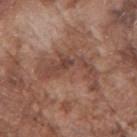Clinical impression: The lesion was photographed on a routine skin check and not biopsied; there is no pathology result. Context: The lesion's longest dimension is about 7 mm. This is a white-light tile. A male patient, aged approximately 75. The lesion-visualizer software estimated a footprint of about 16 mm², a shape eccentricity near 0.85, and a symmetry-axis asymmetry near 0.6. The analysis additionally found a mean CIELAB color near L≈45 a*≈20 b*≈26, about 8 CIELAB-L* units darker than the surrounding skin, and a lesion-to-skin contrast of about 6 (normalized; higher = more distinct). It also reported border irregularity of about 9.5 on a 0–10 scale, internal color variation of about 4 on a 0–10 scale, and peripheral color asymmetry of about 1. It also reported an automated nevus-likeness rating near 0 out of 100 and a detector confidence of about 75 out of 100 that the crop contains a lesion. The lesion is located on the right upper arm. A 15 mm close-up extracted from a 3D total-body photography capture.